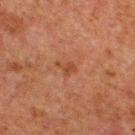<record>
<biopsy_status>not biopsied; imaged during a skin examination</biopsy_status>
<lighting>cross-polarized</lighting>
<lesion_size>
  <long_diameter_mm_approx>2.5</long_diameter_mm_approx>
</lesion_size>
<patient>
  <sex>male</sex>
  <age_approx>60</age_approx>
</patient>
<image>
  <source>total-body photography crop</source>
  <field_of_view_mm>15</field_of_view_mm>
</image>
<site>upper back</site>
</record>